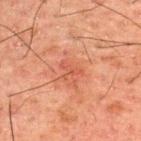Clinical impression: Part of a total-body skin-imaging series; this lesion was reviewed on a skin check and was not flagged for biopsy. Image and clinical context: Cropped from a whole-body photographic skin survey; the tile spans about 15 mm. Captured under cross-polarized illumination. Automated tile analysis of the lesion measured two-axis asymmetry of about 0.65. And it measured a lesion color around L≈43 a*≈26 b*≈29 in CIELAB and a lesion–skin lightness drop of about 6. And it measured a border-irregularity index near 7/10, internal color variation of about 0.5 on a 0–10 scale, and peripheral color asymmetry of about 0. From the upper back. The patient is a male aged around 50.